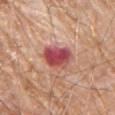No biopsy was performed on this lesion — it was imaged during a full skin examination and was not determined to be concerning. Captured under white-light illumination. The lesion is located on the arm. A male subject roughly 80 years of age. About 4.5 mm across. A close-up tile cropped from a whole-body skin photograph, about 15 mm across.